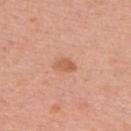follow-up: imaged on a skin check; not biopsied
imaging modality: ~15 mm tile from a whole-body skin photo
tile lighting: white-light
location: the upper back
patient: female, aged 38–42